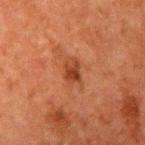Captured during whole-body skin photography for melanoma surveillance; the lesion was not biopsied. A 15 mm close-up tile from a total-body photography series done for melanoma screening. The total-body-photography lesion software estimated a lesion color around L≈33 a*≈24 b*≈30 in CIELAB and roughly 9 lightness units darker than nearby skin. On the right upper arm. The subject is a male aged around 60. The lesion's longest dimension is about 2.5 mm.